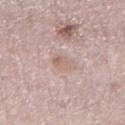Impression:
Part of a total-body skin-imaging series; this lesion was reviewed on a skin check and was not flagged for biopsy.
Background:
The tile uses white-light illumination. Cropped from a total-body skin-imaging series; the visible field is about 15 mm. The recorded lesion diameter is about 3 mm. A male subject aged 73 to 77. From the leg.